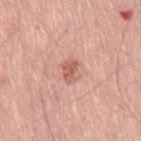The lesion was tiled from a total-body skin photograph and was not biopsied. On the leg. A male patient, aged 68–72. This image is a 15 mm lesion crop taken from a total-body photograph.